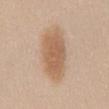{"biopsy_status": "not biopsied; imaged during a skin examination", "lighting": "white-light", "image": {"source": "total-body photography crop", "field_of_view_mm": 15}, "automated_metrics": {"nevus_likeness_0_100": 95}, "patient": {"sex": "female", "age_approx": 40}, "site": "chest"}